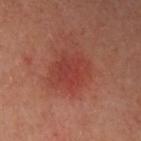follow-up: no biopsy performed (imaged during a skin exam)
subject: male, about 65 years old
location: the left upper arm
imaging modality: ~15 mm tile from a whole-body skin photo
tile lighting: cross-polarized illumination
image-analysis metrics: a mean CIELAB color near L≈40 a*≈30 b*≈28, roughly 6 lightness units darker than nearby skin, and a lesion-to-skin contrast of about 5.5 (normalized; higher = more distinct); a nevus-likeness score of about 85/100 and a lesion-detection confidence of about 100/100
lesion diameter: ≈5 mm